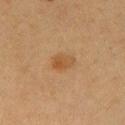A female subject, aged around 55.
The lesion's longest dimension is about 2.5 mm.
A 15 mm close-up extracted from a 3D total-body photography capture.
The lesion is on the left upper arm.
Automated tile analysis of the lesion measured an area of roughly 4.5 mm², an eccentricity of roughly 0.75, and two-axis asymmetry of about 0.2. It also reported an automated nevus-likeness rating near 90 out of 100 and a detector confidence of about 100 out of 100 that the crop contains a lesion.